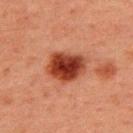biopsy status = catalogued during a skin exam; not biopsied | image source = ~15 mm crop, total-body skin-cancer survey | subject = male, aged approximately 60 | lighting = cross-polarized | body site = the back | size = about 4.5 mm.